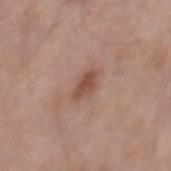Impression: This lesion was catalogued during total-body skin photography and was not selected for biopsy. Background: Captured under white-light illumination. A lesion tile, about 15 mm wide, cut from a 3D total-body photograph. Approximately 3 mm at its widest. Automated tile analysis of the lesion measured border irregularity of about 3 on a 0–10 scale, a within-lesion color-variation index near 2/10, and peripheral color asymmetry of about 0.5. The analysis additionally found a nevus-likeness score of about 35/100 and lesion-presence confidence of about 100/100. A male subject aged approximately 55. Located on the mid back.